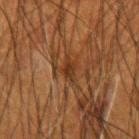Recorded during total-body skin imaging; not selected for excision or biopsy. The lesion is located on the right forearm. Automated image analysis of the tile measured an average lesion color of about L≈27 a*≈18 b*≈27 (CIELAB), roughly 7 lightness units darker than nearby skin, and a lesion-to-skin contrast of about 7.5 (normalized; higher = more distinct). Measured at roughly 2.5 mm in maximum diameter. Cropped from a total-body skin-imaging series; the visible field is about 15 mm. The patient is a male approximately 50 years of age.